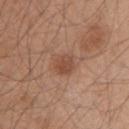Impression: The lesion was photographed on a routine skin check and not biopsied; there is no pathology result. Clinical summary: A roughly 15 mm field-of-view crop from a total-body skin photograph. A male patient roughly 45 years of age. About 3 mm across. From the right upper arm. Automated tile analysis of the lesion measured a lesion area of about 5 mm², a shape eccentricity near 0.55, and two-axis asymmetry of about 0.15. The software also gave a lesion color around L≈48 a*≈22 b*≈30 in CIELAB and a lesion-to-skin contrast of about 6.5 (normalized; higher = more distinct). And it measured border irregularity of about 1.5 on a 0–10 scale, internal color variation of about 2.5 on a 0–10 scale, and a peripheral color-asymmetry measure near 1.